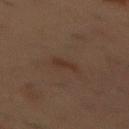No biopsy was performed on this lesion — it was imaged during a full skin examination and was not determined to be concerning. A roughly 15 mm field-of-view crop from a total-body skin photograph. About 2.5 mm across. A male subject, roughly 55 years of age. The lesion is located on the chest. Imaged with cross-polarized lighting.